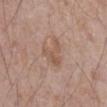{"biopsy_status": "not biopsied; imaged during a skin examination", "lighting": "white-light", "site": "front of the torso", "image": {"source": "total-body photography crop", "field_of_view_mm": 15}, "patient": {"sex": "male", "age_approx": 70}}